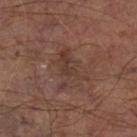The lesion is located on the left lower leg. Imaged with cross-polarized lighting. The recorded lesion diameter is about 5 mm. The patient is a male about 65 years old. Cropped from a whole-body photographic skin survey; the tile spans about 15 mm.